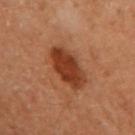Q: Was a biopsy performed?
A: total-body-photography surveillance lesion; no biopsy